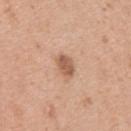{"biopsy_status": "not biopsied; imaged during a skin examination", "image": {"source": "total-body photography crop", "field_of_view_mm": 15}, "lesion_size": {"long_diameter_mm_approx": 2.5}, "site": "left upper arm", "patient": {"sex": "female", "age_approx": 40}, "lighting": "white-light"}